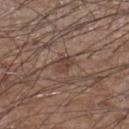workup=total-body-photography surveillance lesion; no biopsy | patient=male, aged 58–62 | image source=15 mm crop, total-body photography | anatomic site=the right lower leg | diameter=~2.5 mm (longest diameter).